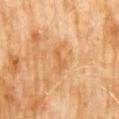Impression:
The lesion was tiled from a total-body skin photograph and was not biopsied.
Background:
Cropped from a whole-body photographic skin survey; the tile spans about 15 mm. The lesion is located on the abdomen. A male patient, aged approximately 60.